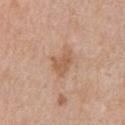workup = total-body-photography surveillance lesion; no biopsy
acquisition = total-body-photography crop, ~15 mm field of view
lesion size = about 3 mm
location = the abdomen
subject = male, approximately 70 years of age